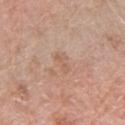Case summary:
- workup: catalogued during a skin exam; not biopsied